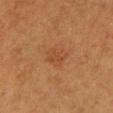Captured during whole-body skin photography for melanoma surveillance; the lesion was not biopsied. Located on the front of the torso. A 15 mm crop from a total-body photograph taken for skin-cancer surveillance. A female subject, about 40 years old. Captured under cross-polarized illumination.